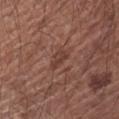notes: catalogued during a skin exam; not biopsied
subject: male, in their mid-60s
anatomic site: the left upper arm
image source: ~15 mm tile from a whole-body skin photo